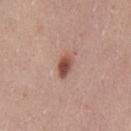Assessment:
This lesion was catalogued during total-body skin photography and was not selected for biopsy.
Image and clinical context:
Captured under white-light illumination. Located on the front of the torso. About 3 mm across. The patient is a male aged 23–27. A region of skin cropped from a whole-body photographic capture, roughly 15 mm wide.